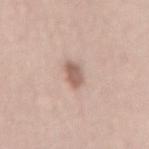Part of a total-body skin-imaging series; this lesion was reviewed on a skin check and was not flagged for biopsy.
The recorded lesion diameter is about 3.5 mm.
The lesion is on the mid back.
Automated image analysis of the tile measured about 13 CIELAB-L* units darker than the surrounding skin and a lesion-to-skin contrast of about 8 (normalized; higher = more distinct).
The subject is a female in their mid-60s.
A lesion tile, about 15 mm wide, cut from a 3D total-body photograph.